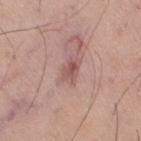| field | value |
|---|---|
| size | ~3 mm (longest diameter) |
| illumination | white-light illumination |
| anatomic site | the right thigh |
| image source | ~15 mm crop, total-body skin-cancer survey |
| TBP lesion metrics | a mean CIELAB color near L≈54 a*≈22 b*≈23, roughly 9 lightness units darker than nearby skin, and a normalized lesion–skin contrast near 6.5; a peripheral color-asymmetry measure near 1.5 |
| patient | male, about 60 years old |